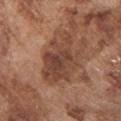A male patient, in their mid- to late 70s. Approximately 6.5 mm at its widest. The lesion is located on the chest. Imaged with white-light lighting. An algorithmic analysis of the crop reported a lesion area of about 19 mm², an outline eccentricity of about 0.75 (0 = round, 1 = elongated), and a shape-asymmetry score of about 0.25 (0 = symmetric). The analysis additionally found a mean CIELAB color near L≈43 a*≈21 b*≈28 and about 11 CIELAB-L* units darker than the surrounding skin. The analysis additionally found a nevus-likeness score of about 5/100 and lesion-presence confidence of about 100/100. A lesion tile, about 15 mm wide, cut from a 3D total-body photograph.